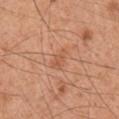subject = male, approximately 60 years of age
lighting = white-light
location = the right upper arm
acquisition = ~15 mm tile from a whole-body skin photo